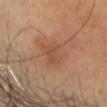Q: Is there a histopathology result?
A: total-body-photography surveillance lesion; no biopsy
Q: Where on the body is the lesion?
A: the head or neck
Q: What are the patient's age and sex?
A: male, in their mid-50s
Q: What kind of image is this?
A: ~15 mm tile from a whole-body skin photo